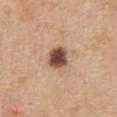Clinical impression: The lesion was tiled from a total-body skin photograph and was not biopsied. Background: A female patient approximately 45 years of age. The tile uses white-light illumination. The total-body-photography lesion software estimated a footprint of about 7 mm², an eccentricity of roughly 0.4, and a shape-asymmetry score of about 0.15 (0 = symmetric). A 15 mm crop from a total-body photograph taken for skin-cancer surveillance. On the abdomen.